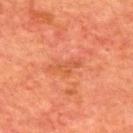<lesion>
<biopsy_status>not biopsied; imaged during a skin examination</biopsy_status>
<image>
  <source>total-body photography crop</source>
  <field_of_view_mm>15</field_of_view_mm>
</image>
<patient>
  <sex>male</sex>
  <age_approx>65</age_approx>
</patient>
<site>upper back</site>
</lesion>